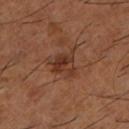Q: Is there a histopathology result?
A: catalogued during a skin exam; not biopsied
Q: What kind of image is this?
A: ~15 mm crop, total-body skin-cancer survey
Q: What are the patient's age and sex?
A: male, roughly 65 years of age
Q: Automated lesion metrics?
A: about 8 CIELAB-L* units darker than the surrounding skin and a normalized lesion–skin contrast near 7.5; a border-irregularity rating of about 3.5/10, internal color variation of about 5 on a 0–10 scale, and radial color variation of about 2; a classifier nevus-likeness of about 30/100 and a detector confidence of about 100 out of 100 that the crop contains a lesion
Q: What is the anatomic site?
A: the left lower leg
Q: What lighting was used for the tile?
A: cross-polarized illumination